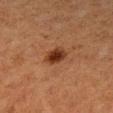biopsy status: catalogued during a skin exam; not biopsied | subject: female, aged approximately 40 | image source: total-body-photography crop, ~15 mm field of view | lighting: cross-polarized illumination | anatomic site: the right forearm | size: ~2.5 mm (longest diameter).